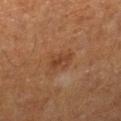workup=no biopsy performed (imaged during a skin exam) | automated metrics=a classifier nevus-likeness of about 5/100 | diameter=≈3 mm | image=~15 mm tile from a whole-body skin photo | patient=male, aged 58–62 | illumination=cross-polarized illumination | body site=the leg.